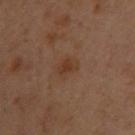The lesion was photographed on a routine skin check and not biopsied; there is no pathology result.